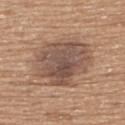This lesion was catalogued during total-body skin photography and was not selected for biopsy. A male patient, aged around 65. A 15 mm crop from a total-body photograph taken for skin-cancer surveillance. The recorded lesion diameter is about 7 mm. The lesion-visualizer software estimated a mean CIELAB color near L≈51 a*≈17 b*≈26 and about 11 CIELAB-L* units darker than the surrounding skin. The software also gave a border-irregularity index near 3/10, a color-variation rating of about 5.5/10, and peripheral color asymmetry of about 2. The software also gave a classifier nevus-likeness of about 35/100 and a lesion-detection confidence of about 100/100. This is a white-light tile. On the upper back.